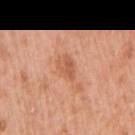This lesion was catalogued during total-body skin photography and was not selected for biopsy. On the right upper arm. A female subject, aged 38–42. A close-up tile cropped from a whole-body skin photograph, about 15 mm across.